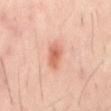Q: Is there a histopathology result?
A: catalogued during a skin exam; not biopsied
Q: Illumination type?
A: cross-polarized illumination
Q: Automated lesion metrics?
A: a border-irregularity index near 2.5/10 and internal color variation of about 3.5 on a 0–10 scale; a lesion-detection confidence of about 100/100
Q: What is the anatomic site?
A: the mid back
Q: What kind of image is this?
A: ~15 mm tile from a whole-body skin photo
Q: What are the patient's age and sex?
A: male, aged 38–42